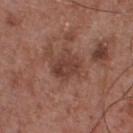{"lesion_size": {"long_diameter_mm_approx": 3.5}, "site": "front of the torso", "image": {"source": "total-body photography crop", "field_of_view_mm": 15}, "lighting": "white-light", "patient": {"sex": "male", "age_approx": 55}, "automated_metrics": {"cielab_L": 40, "cielab_a": 21, "cielab_b": 25, "vs_skin_contrast_norm": 7.0, "nevus_likeness_0_100": 0, "lesion_detection_confidence_0_100": 100}}